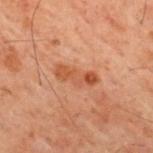Imaged during a routine full-body skin examination; the lesion was not biopsied and no histopathology is available. From the upper back. Approximately 4.5 mm at its widest. An algorithmic analysis of the crop reported a within-lesion color-variation index near 4.5/10 and peripheral color asymmetry of about 1. The tile uses cross-polarized illumination. A male subject about 60 years old. A region of skin cropped from a whole-body photographic capture, roughly 15 mm wide.